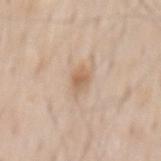notes: no biopsy performed (imaged during a skin exam)
illumination: white-light
patient: male, aged around 60
lesion diameter: ≈3 mm
anatomic site: the mid back
image: total-body-photography crop, ~15 mm field of view